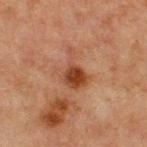Q: Is there a histopathology result?
A: imaged on a skin check; not biopsied
Q: Automated lesion metrics?
A: a footprint of about 9.5 mm², an eccentricity of roughly 0.7, and a shape-asymmetry score of about 0.35 (0 = symmetric); an average lesion color of about L≈36 a*≈21 b*≈28 (CIELAB), about 9 CIELAB-L* units darker than the surrounding skin, and a normalized lesion–skin contrast near 8; a border-irregularity rating of about 5/10, a within-lesion color-variation index near 5.5/10, and peripheral color asymmetry of about 1.5; a classifier nevus-likeness of about 90/100 and lesion-presence confidence of about 100/100
Q: Lesion size?
A: ~4.5 mm (longest diameter)
Q: How was the tile lit?
A: cross-polarized
Q: Where on the body is the lesion?
A: the front of the torso
Q: What are the patient's age and sex?
A: male, roughly 65 years of age
Q: What kind of image is this?
A: ~15 mm tile from a whole-body skin photo